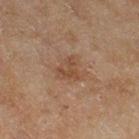| key | value |
|---|---|
| follow-up | no biopsy performed (imaged during a skin exam) |
| image | 15 mm crop, total-body photography |
| subject | female, aged approximately 60 |
| body site | the right thigh |